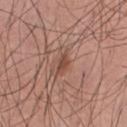notes = total-body-photography surveillance lesion; no biopsy
acquisition = 15 mm crop, total-body photography
anatomic site = the chest
subject = male, aged 53–57
size = ≈2.5 mm